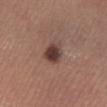<record>
<biopsy_status>not biopsied; imaged during a skin examination</biopsy_status>
<image>
  <source>total-body photography crop</source>
  <field_of_view_mm>15</field_of_view_mm>
</image>
<automated_metrics>
  <border_irregularity_0_10>2.0</border_irregularity_0_10>
  <color_variation_0_10>3.0</color_variation_0_10>
  <peripheral_color_asymmetry>1.0</peripheral_color_asymmetry>
  <nevus_likeness_0_100>95</nevus_likeness_0_100>
  <lesion_detection_confidence_0_100>100</lesion_detection_confidence_0_100>
</automated_metrics>
<site>right lower leg</site>
<lesion_size>
  <long_diameter_mm_approx>3.0</long_diameter_mm_approx>
</lesion_size>
<lighting>white-light</lighting>
<patient>
  <sex>male</sex>
  <age_approx>70</age_approx>
</patient>
</record>